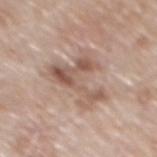Recorded during total-body skin imaging; not selected for excision or biopsy. A 15 mm close-up extracted from a 3D total-body photography capture. A male subject, aged approximately 80. From the mid back.